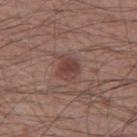<case>
  <biopsy_status>not biopsied; imaged during a skin examination</biopsy_status>
</case>